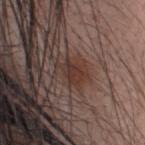{"biopsy_status": "not biopsied; imaged during a skin examination", "patient": {"sex": "male", "age_approx": 35}, "image": {"source": "total-body photography crop", "field_of_view_mm": 15}, "automated_metrics": {"border_irregularity_0_10": 4.5, "color_variation_0_10": 4.0, "peripheral_color_asymmetry": 1.5}, "lesion_size": {"long_diameter_mm_approx": 3.5}, "site": "head or neck"}